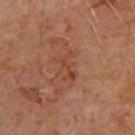No biopsy was performed on this lesion — it was imaged during a full skin examination and was not determined to be concerning. The lesion's longest dimension is about 3.5 mm. A 15 mm close-up extracted from a 3D total-body photography capture. The lesion is located on the upper back. A male subject, aged around 65.